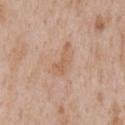Q: What are the patient's age and sex?
A: male, aged 63 to 67
Q: How was this image acquired?
A: total-body-photography crop, ~15 mm field of view
Q: Lesion location?
A: the chest